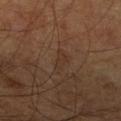Part of a total-body skin-imaging series; this lesion was reviewed on a skin check and was not flagged for biopsy. This is a cross-polarized tile. The patient is a male aged 63–67. From the leg. A lesion tile, about 15 mm wide, cut from a 3D total-body photograph. The recorded lesion diameter is about 3 mm.